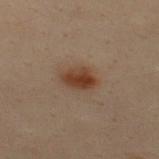Impression:
Recorded during total-body skin imaging; not selected for excision or biopsy.
Image and clinical context:
A male subject, aged around 30. A roughly 15 mm field-of-view crop from a total-body skin photograph. From the upper back.